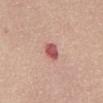notes=imaged on a skin check; not biopsied | lesion size=≈2.5 mm | patient=female, aged 53 to 57 | illumination=white-light | location=the abdomen | image=15 mm crop, total-body photography.